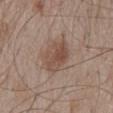This lesion was catalogued during total-body skin photography and was not selected for biopsy.
An algorithmic analysis of the crop reported an area of roughly 11 mm², an eccentricity of roughly 0.85, and a shape-asymmetry score of about 0.15 (0 = symmetric). It also reported a mean CIELAB color near L≈49 a*≈17 b*≈25, roughly 9 lightness units darker than nearby skin, and a lesion-to-skin contrast of about 7 (normalized; higher = more distinct). It also reported border irregularity of about 2 on a 0–10 scale and radial color variation of about 2. And it measured an automated nevus-likeness rating near 70 out of 100 and a lesion-detection confidence of about 100/100.
The subject is a male roughly 55 years of age.
Imaged with white-light lighting.
A region of skin cropped from a whole-body photographic capture, roughly 15 mm wide.
From the mid back.
The lesion's longest dimension is about 5 mm.